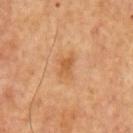workup: total-body-photography surveillance lesion; no biopsy | image source: 15 mm crop, total-body photography | lesion diameter: ~3 mm (longest diameter) | subject: male, approximately 65 years of age.